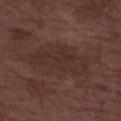Findings:
• subject: male, aged 73–77
• tile lighting: white-light illumination
• body site: the right forearm
• image source: ~15 mm tile from a whole-body skin photo